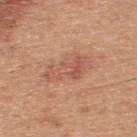{
  "biopsy_status": "not biopsied; imaged during a skin examination",
  "patient": {
    "sex": "male",
    "age_approx": 45
  },
  "site": "upper back",
  "image": {
    "source": "total-body photography crop",
    "field_of_view_mm": 15
  }
}